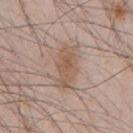biopsy status — catalogued during a skin exam; not biopsied | lesion diameter — ~5 mm (longest diameter) | patient — male, aged 43–47 | image source — ~15 mm tile from a whole-body skin photo | location — the chest | illumination — white-light.